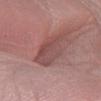A lesion tile, about 15 mm wide, cut from a 3D total-body photograph. A male subject, about 55 years old.